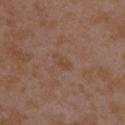• imaging modality — ~15 mm tile from a whole-body skin photo
• image-analysis metrics — border irregularity of about 4 on a 0–10 scale, a within-lesion color-variation index near 0/10, and radial color variation of about 0; an automated nevus-likeness rating near 0 out of 100 and a lesion-detection confidence of about 100/100
• size — ~2.5 mm (longest diameter)
• illumination — white-light
• subject — female, roughly 35 years of age
• location — the right upper arm